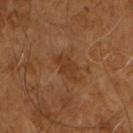Assessment: Part of a total-body skin-imaging series; this lesion was reviewed on a skin check and was not flagged for biopsy. Acquisition and patient details: Automated tile analysis of the lesion measured border irregularity of about 3.5 on a 0–10 scale, a within-lesion color-variation index near 2/10, and a peripheral color-asymmetry measure near 1. And it measured a detector confidence of about 100 out of 100 that the crop contains a lesion. The patient is a male aged around 65. The tile uses cross-polarized illumination. A 15 mm close-up tile from a total-body photography series done for melanoma screening.